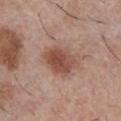automated metrics = a border-irregularity rating of about 2.5/10, internal color variation of about 4.5 on a 0–10 scale, and a peripheral color-asymmetry measure near 1.5; a nevus-likeness score of about 95/100 | tile lighting = white-light | subject = male, aged approximately 30 | diameter = ~4.5 mm (longest diameter) | image source = 15 mm crop, total-body photography | site = the chest.